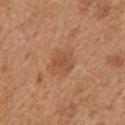{"biopsy_status": "not biopsied; imaged during a skin examination", "site": "left upper arm", "patient": {"sex": "female", "age_approx": 40}, "image": {"source": "total-body photography crop", "field_of_view_mm": 15}}